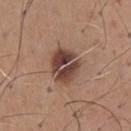Assessment:
Part of a total-body skin-imaging series; this lesion was reviewed on a skin check and was not flagged for biopsy.
Clinical summary:
A region of skin cropped from a whole-body photographic capture, roughly 15 mm wide. The lesion is located on the chest. About 4.5 mm across. A male subject aged around 65.